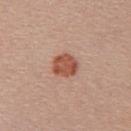| key | value |
|---|---|
| follow-up | no biopsy performed (imaged during a skin exam) |
| lighting | white-light illumination |
| imaging modality | 15 mm crop, total-body photography |
| site | the upper back |
| subject | female, about 25 years old |
| lesion diameter | ≈3 mm |